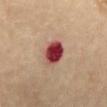Assessment: No biopsy was performed on this lesion — it was imaged during a full skin examination and was not determined to be concerning. Image and clinical context: The lesion is on the front of the torso. This image is a 15 mm lesion crop taken from a total-body photograph. A female patient roughly 70 years of age.